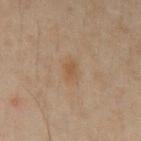The lesion was photographed on a routine skin check and not biopsied; there is no pathology result. From the chest. A male patient, roughly 50 years of age. Captured under cross-polarized illumination. A roughly 15 mm field-of-view crop from a total-body skin photograph. The recorded lesion diameter is about 2.5 mm. Automated image analysis of the tile measured a lesion area of about 3 mm², a shape eccentricity near 0.85, and a shape-asymmetry score of about 0.35 (0 = symmetric). And it measured an automated nevus-likeness rating near 75 out of 100 and a lesion-detection confidence of about 100/100.